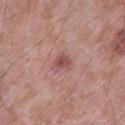Impression:
Captured during whole-body skin photography for melanoma surveillance; the lesion was not biopsied.
Acquisition and patient details:
Measured at roughly 2.5 mm in maximum diameter. The lesion is located on the left lower leg. A 15 mm close-up tile from a total-body photography series done for melanoma screening. Captured under white-light illumination. A male subject aged approximately 55. The total-body-photography lesion software estimated an area of roughly 4.5 mm², an outline eccentricity of about 0.35 (0 = round, 1 = elongated), and a shape-asymmetry score of about 0.35 (0 = symmetric). And it measured an average lesion color of about L≈52 a*≈24 b*≈23 (CIELAB), a lesion–skin lightness drop of about 9, and a normalized lesion–skin contrast near 6.5. The software also gave a border-irregularity index near 3/10, internal color variation of about 3 on a 0–10 scale, and peripheral color asymmetry of about 1. The software also gave a classifier nevus-likeness of about 5/100 and lesion-presence confidence of about 100/100.